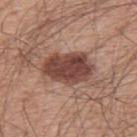Assessment:
This lesion was catalogued during total-body skin photography and was not selected for biopsy.
Context:
A male patient aged around 55. A roughly 15 mm field-of-view crop from a total-body skin photograph. Located on the upper back. The lesion's longest dimension is about 6 mm. This is a white-light tile.